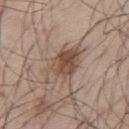Notes:
– biopsy status: total-body-photography surveillance lesion; no biopsy
– TBP lesion metrics: a lesion color around L≈50 a*≈16 b*≈27 in CIELAB; border irregularity of about 2.5 on a 0–10 scale and a within-lesion color-variation index near 7.5/10
– patient: male, aged 53 to 57
– tile lighting: white-light illumination
– size: about 6 mm
– acquisition: total-body-photography crop, ~15 mm field of view
– body site: the mid back